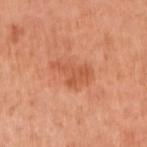workup: total-body-photography surveillance lesion; no biopsy | site: the left arm | subject: female, aged around 65 | image: ~15 mm tile from a whole-body skin photo | lighting: white-light illumination | automated lesion analysis: an outline eccentricity of about 0.8 (0 = round, 1 = elongated) and a shape-asymmetry score of about 0.45 (0 = symmetric); border irregularity of about 5 on a 0–10 scale and internal color variation of about 2.5 on a 0–10 scale; a classifier nevus-likeness of about 5/100.